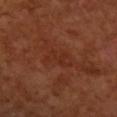| key | value |
|---|---|
| follow-up | no biopsy performed (imaged during a skin exam) |
| body site | the right forearm |
| image source | ~15 mm tile from a whole-body skin photo |
| patient | female, about 50 years old |
| lighting | cross-polarized |
| image-analysis metrics | an area of roughly 4.5 mm² and a symmetry-axis asymmetry near 0.55; border irregularity of about 7.5 on a 0–10 scale, a color-variation rating of about 0/10, and a peripheral color-asymmetry measure near 0; a classifier nevus-likeness of about 0/100 and lesion-presence confidence of about 100/100 |